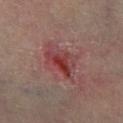Assessment: This lesion was catalogued during total-body skin photography and was not selected for biopsy. Clinical summary: Automated tile analysis of the lesion measured a footprint of about 9.5 mm², an eccentricity of roughly 0.7, and a symmetry-axis asymmetry near 0.3. Cropped from a total-body skin-imaging series; the visible field is about 15 mm. Imaged with cross-polarized lighting. Located on the right lower leg. The lesion's longest dimension is about 4 mm. A male subject, in their mid-50s.